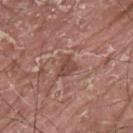Clinical impression:
Part of a total-body skin-imaging series; this lesion was reviewed on a skin check and was not flagged for biopsy.
Image and clinical context:
This image is a 15 mm lesion crop taken from a total-body photograph. A male patient in their 40s. Approximately 2.5 mm at its widest. From the upper back.